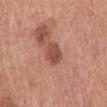Assessment: The lesion was tiled from a total-body skin photograph and was not biopsied. Acquisition and patient details: The lesion is on the mid back. The total-body-photography lesion software estimated a mean CIELAB color near L≈49 a*≈25 b*≈27. It also reported an automated nevus-likeness rating near 55 out of 100 and a lesion-detection confidence of about 100/100. A region of skin cropped from a whole-body photographic capture, roughly 15 mm wide. This is a white-light tile. About 3.5 mm across. The patient is a male aged approximately 70.